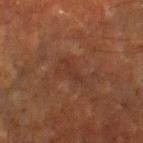Part of a total-body skin-imaging series; this lesion was reviewed on a skin check and was not flagged for biopsy. The lesion's longest dimension is about 3.5 mm. A male subject aged around 65. Cropped from a total-body skin-imaging series; the visible field is about 15 mm. The total-body-photography lesion software estimated a border-irregularity index near 7/10 and radial color variation of about 0. And it measured a lesion-detection confidence of about 95/100. The lesion is located on the right lower leg.